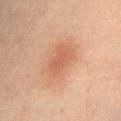notes = imaged on a skin check; not biopsied
site = the lower back
illumination = cross-polarized
subject = male, approximately 65 years of age
image source = ~15 mm crop, total-body skin-cancer survey
lesion diameter = about 5 mm
automated metrics = two-axis asymmetry of about 0.25; a classifier nevus-likeness of about 65/100 and lesion-presence confidence of about 100/100